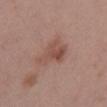  biopsy_status: not biopsied; imaged during a skin examination
  lighting: white-light
  site: chest
  patient:
    sex: female
    age_approx: 40
  image:
    source: total-body photography crop
    field_of_view_mm: 15